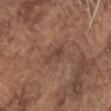notes=imaged on a skin check; not biopsied | automated metrics=a color-variation rating of about 1.5/10 | lighting=white-light | anatomic site=the head or neck | subject=male, in their mid-70s | size=≈2.5 mm | image=~15 mm crop, total-body skin-cancer survey.